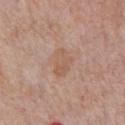workup: no biopsy performed (imaged during a skin exam)
subject: male, aged around 60
image: ~15 mm tile from a whole-body skin photo
tile lighting: white-light illumination
anatomic site: the front of the torso